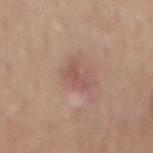Q: Was a biopsy performed?
A: no biopsy performed (imaged during a skin exam)
Q: Lesion size?
A: ≈2.5 mm
Q: How was this image acquired?
A: ~15 mm tile from a whole-body skin photo
Q: Where on the body is the lesion?
A: the back
Q: Patient demographics?
A: male, about 50 years old